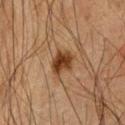The lesion was tiled from a total-body skin photograph and was not biopsied. The lesion is located on the chest. Cropped from a whole-body photographic skin survey; the tile spans about 15 mm. Measured at roughly 3 mm in maximum diameter. A male patient, about 65 years old. Automated tile analysis of the lesion measured a mean CIELAB color near L≈32 a*≈19 b*≈30, about 13 CIELAB-L* units darker than the surrounding skin, and a lesion-to-skin contrast of about 11.5 (normalized; higher = more distinct). It also reported a border-irregularity rating of about 2.5/10, a within-lesion color-variation index near 3.5/10, and radial color variation of about 1.5. The tile uses cross-polarized illumination.